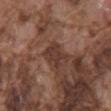Clinical impression: No biopsy was performed on this lesion — it was imaged during a full skin examination and was not determined to be concerning. Background: An algorithmic analysis of the crop reported an area of roughly 6.5 mm² and a symmetry-axis asymmetry near 0.35. And it measured border irregularity of about 4.5 on a 0–10 scale and a within-lesion color-variation index near 2/10. The software also gave a nevus-likeness score of about 0/100 and a detector confidence of about 95 out of 100 that the crop contains a lesion. The tile uses white-light illumination. The lesion is located on the mid back. Cropped from a total-body skin-imaging series; the visible field is about 15 mm. The lesion's longest dimension is about 3.5 mm. A male patient, in their mid- to late 70s.